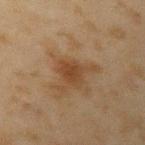biopsy status: no biopsy performed (imaged during a skin exam)
image source: 15 mm crop, total-body photography
lighting: cross-polarized illumination
site: the left upper arm
patient: male, approximately 45 years of age